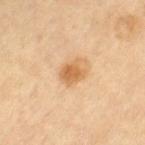Imaged during a routine full-body skin examination; the lesion was not biopsied and no histopathology is available. A 15 mm close-up tile from a total-body photography series done for melanoma screening. The lesion is on the left thigh. The subject is a female aged 68–72.